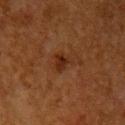No biopsy was performed on this lesion — it was imaged during a full skin examination and was not determined to be concerning. A 15 mm crop from a total-body photograph taken for skin-cancer surveillance. A female subject aged around 50. Longest diameter approximately 2.5 mm. From the left upper arm.